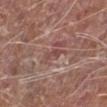Q: Was a biopsy performed?
A: no biopsy performed (imaged during a skin exam)
Q: Patient demographics?
A: male, aged approximately 80
Q: What lighting was used for the tile?
A: white-light illumination
Q: What did automated image analysis measure?
A: a lesion color around L≈46 a*≈22 b*≈23 in CIELAB, about 8 CIELAB-L* units darker than the surrounding skin, and a normalized lesion–skin contrast near 6.5; a border-irregularity index near 3.5/10, a color-variation rating of about 0/10, and peripheral color asymmetry of about 0; a nevus-likeness score of about 0/100 and lesion-presence confidence of about 60/100
Q: How large is the lesion?
A: about 2.5 mm
Q: Lesion location?
A: the left lower leg
Q: What kind of image is this?
A: 15 mm crop, total-body photography